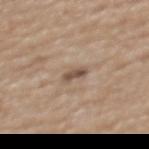<record>
  <biopsy_status>not biopsied; imaged during a skin examination</biopsy_status>
  <patient>
    <sex>female</sex>
    <age_approx>70</age_approx>
  </patient>
  <lesion_size>
    <long_diameter_mm_approx>2.5</long_diameter_mm_approx>
  </lesion_size>
  <lighting>white-light</lighting>
  <image>
    <source>total-body photography crop</source>
    <field_of_view_mm>15</field_of_view_mm>
  </image>
  <site>back</site>
</record>